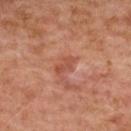  biopsy_status: not biopsied; imaged during a skin examination
  lighting: cross-polarized
  image:
    source: total-body photography crop
    field_of_view_mm: 15
  patient:
    sex: female
    age_approx: 60
  lesion_size:
    long_diameter_mm_approx: 2.5
  site: upper back